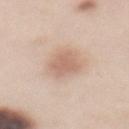| key | value |
|---|---|
| image-analysis metrics | a lesion area of about 8.5 mm², a shape eccentricity near 0.8, and two-axis asymmetry of about 0.15; a lesion color around L≈64 a*≈19 b*≈28 in CIELAB, a lesion–skin lightness drop of about 10, and a lesion-to-skin contrast of about 6.5 (normalized; higher = more distinct); a border-irregularity index near 2/10, a within-lesion color-variation index near 1.5/10, and radial color variation of about 0.5; a classifier nevus-likeness of about 90/100 and a detector confidence of about 100 out of 100 that the crop contains a lesion |
| image | 15 mm crop, total-body photography |
| tile lighting | white-light |
| lesion size | about 4.5 mm |
| subject | female, aged 48–52 |
| anatomic site | the mid back |